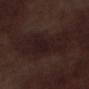biopsy_status: not biopsied; imaged during a skin examination
patient:
  sex: male
  age_approx: 70
lighting: white-light
lesion_size:
  long_diameter_mm_approx: 8.0
automated_metrics:
  area_mm2_approx: 20.0
  shape_asymmetry: 0.4
  border_irregularity_0_10: 5.5
  peripheral_color_asymmetry: 1.0
image:
  source: total-body photography crop
  field_of_view_mm: 15
site: left lower leg